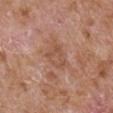Impression:
This lesion was catalogued during total-body skin photography and was not selected for biopsy.
Image and clinical context:
This is a white-light tile. A male subject roughly 65 years of age. On the right upper arm. An algorithmic analysis of the crop reported a shape eccentricity near 0.75 and two-axis asymmetry of about 0.4. And it measured border irregularity of about 3.5 on a 0–10 scale, internal color variation of about 3 on a 0–10 scale, and peripheral color asymmetry of about 1. The software also gave a nevus-likeness score of about 0/100 and a lesion-detection confidence of about 100/100. A 15 mm close-up extracted from a 3D total-body photography capture.